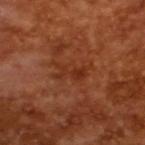<lesion>
  <biopsy_status>not biopsied; imaged during a skin examination</biopsy_status>
  <automated_metrics>
    <area_mm2_approx>4.0</area_mm2_approx>
    <eccentricity>0.9</eccentricity>
    <shape_asymmetry>0.45</shape_asymmetry>
    <cielab_L>31</cielab_L>
    <cielab_a>27</cielab_a>
    <cielab_b>33</cielab_b>
    <vs_skin_darker_L>6.0</vs_skin_darker_L>
    <border_irregularity_0_10>6.5</border_irregularity_0_10>
    <color_variation_0_10>1.0</color_variation_0_10>
    <peripheral_color_asymmetry>0.0</peripheral_color_asymmetry>
  </automated_metrics>
  <image>
    <source>total-body photography crop</source>
    <field_of_view_mm>15</field_of_view_mm>
  </image>
  <patient>
    <sex>male</sex>
    <age_approx>65</age_approx>
  </patient>
  <lighting>cross-polarized</lighting>
</lesion>